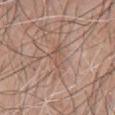lesion_size:
  long_diameter_mm_approx: 4.5
site: chest
lighting: white-light
automated_metrics:
  area_mm2_approx: 6.0
  eccentricity: 0.95
  shape_asymmetry: 0.4
  border_irregularity_0_10: 5.0
  color_variation_0_10: 3.0
  peripheral_color_asymmetry: 1.0
patient:
  sex: male
  age_approx: 70
image:
  source: total-body photography crop
  field_of_view_mm: 15